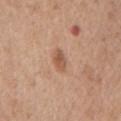<case>
<biopsy_status>not biopsied; imaged during a skin examination</biopsy_status>
<patient>
  <sex>female</sex>
  <age_approx>75</age_approx>
</patient>
<site>abdomen</site>
<image>
  <source>total-body photography crop</source>
  <field_of_view_mm>15</field_of_view_mm>
</image>
</case>